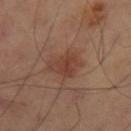Case summary:
- follow-up: catalogued during a skin exam; not biopsied
- anatomic site: the right thigh
- image source: 15 mm crop, total-body photography